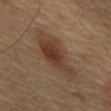follow-up = total-body-photography surveillance lesion; no biopsy
anatomic site = the mid back
subject = male, approximately 65 years of age
imaging modality = ~15 mm tile from a whole-body skin photo
illumination = cross-polarized illumination
size = ~8.5 mm (longest diameter)